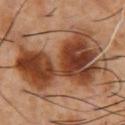The lesion was photographed on a routine skin check and not biopsied; there is no pathology result. The subject is a male aged approximately 55. Located on the chest. Automated tile analysis of the lesion measured an area of roughly 50 mm², an eccentricity of roughly 0.85, and two-axis asymmetry of about 0.35. It also reported a border-irregularity rating of about 6.5/10, a within-lesion color-variation index near 9.5/10, and radial color variation of about 3.5. The software also gave a nevus-likeness score of about 85/100 and a detector confidence of about 100 out of 100 that the crop contains a lesion. The tile uses cross-polarized illumination. A region of skin cropped from a whole-body photographic capture, roughly 15 mm wide. The recorded lesion diameter is about 12 mm.